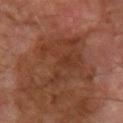Findings:
* notes: imaged on a skin check; not biopsied
* tile lighting: cross-polarized illumination
* patient: male, aged around 70
* lesion size: ≈7 mm
* body site: the right lower leg
* acquisition: ~15 mm crop, total-body skin-cancer survey